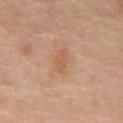Findings:
– biopsy status — no biopsy performed (imaged during a skin exam)
– tile lighting — cross-polarized
– image — total-body-photography crop, ~15 mm field of view
– lesion diameter — ≈3.5 mm
– patient — male, aged around 60
– site — the abdomen